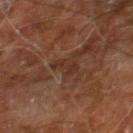follow-up = no biopsy performed (imaged during a skin exam) | diameter = ≈3 mm | lighting = cross-polarized | subject = male, about 60 years old | site = the right leg | image source = ~15 mm crop, total-body skin-cancer survey.